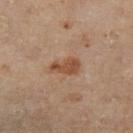This lesion was catalogued during total-body skin photography and was not selected for biopsy. The tile uses cross-polarized illumination. The lesion is on the left lower leg. A female patient aged 58 to 62. The lesion's longest dimension is about 3.5 mm. A 15 mm crop from a total-body photograph taken for skin-cancer surveillance.